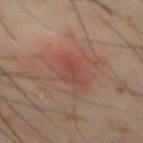  biopsy_status: not biopsied; imaged during a skin examination
  site: mid back
  image:
    source: total-body photography crop
    field_of_view_mm: 15
  lesion_size:
    long_diameter_mm_approx: 4.0
  lighting: cross-polarized
  patient:
    sex: male
    age_approx: 70